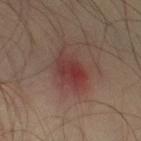Recorded during total-body skin imaging; not selected for excision or biopsy. A male subject in their mid- to late 30s. The recorded lesion diameter is about 4 mm. Located on the left thigh. Cropped from a total-body skin-imaging series; the visible field is about 15 mm.